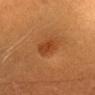Q: How large is the lesion?
A: ~2.5 mm (longest diameter)
Q: What lighting was used for the tile?
A: cross-polarized illumination
Q: What is the imaging modality?
A: ~15 mm crop, total-body skin-cancer survey
Q: What are the patient's age and sex?
A: female, in their 30s
Q: Lesion location?
A: the head or neck